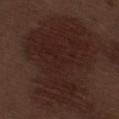workup — imaged on a skin check; not biopsied | image source — total-body-photography crop, ~15 mm field of view | automated lesion analysis — a footprint of about 60 mm², an outline eccentricity of about 0.75 (0 = round, 1 = elongated), and a symmetry-axis asymmetry near 0.5; roughly 6 lightness units darker than nearby skin and a lesion-to-skin contrast of about 7.5 (normalized; higher = more distinct); a within-lesion color-variation index near 2.5/10 and a peripheral color-asymmetry measure near 1 | subject — male, roughly 70 years of age | body site — the leg | tile lighting — white-light illumination.